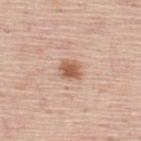The lesion was photographed on a routine skin check and not biopsied; there is no pathology result. This image is a 15 mm lesion crop taken from a total-body photograph. Captured under white-light illumination. A male subject aged approximately 45. On the back. Approximately 2.5 mm at its widest.